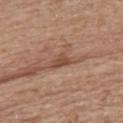Imaged during a routine full-body skin examination; the lesion was not biopsied and no histopathology is available. The tile uses white-light illumination. From the upper back. About 2.5 mm across. The subject is a female aged 68–72. A 15 mm close-up extracted from a 3D total-body photography capture. Automated image analysis of the tile measured a lesion area of about 3 mm², an outline eccentricity of about 0.8 (0 = round, 1 = elongated), and two-axis asymmetry of about 0.4. It also reported a mean CIELAB color near L≈47 a*≈21 b*≈30, a lesion–skin lightness drop of about 10, and a normalized lesion–skin contrast near 7.5. The software also gave a border-irregularity index near 4/10. It also reported a classifier nevus-likeness of about 0/100 and a detector confidence of about 80 out of 100 that the crop contains a lesion.